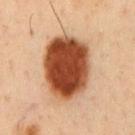Q: Is there a histopathology result?
A: no biopsy performed (imaged during a skin exam)
Q: Patient demographics?
A: male, aged 48 to 52
Q: How was this image acquired?
A: total-body-photography crop, ~15 mm field of view
Q: Automated lesion metrics?
A: a border-irregularity rating of about 1/10, a within-lesion color-variation index near 7.5/10, and peripheral color asymmetry of about 2.5; a lesion-detection confidence of about 100/100
Q: Illumination type?
A: cross-polarized illumination
Q: How large is the lesion?
A: about 7.5 mm
Q: Where on the body is the lesion?
A: the chest